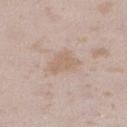follow-up=catalogued during a skin exam; not biopsied
lesion size=about 3.5 mm
site=the leg
lighting=white-light
image=15 mm crop, total-body photography
subject=female, approximately 25 years of age